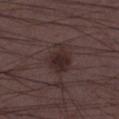| field | value |
|---|---|
| patient | male, aged approximately 50 |
| image | 15 mm crop, total-body photography |
| anatomic site | the right lower leg |
| automated metrics | roughly 8 lightness units darker than nearby skin; a border-irregularity rating of about 4/10 and a within-lesion color-variation index near 2.5/10; an automated nevus-likeness rating near 65 out of 100 |
| illumination | white-light illumination |